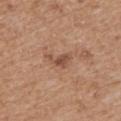Impression:
Captured during whole-body skin photography for melanoma surveillance; the lesion was not biopsied.
Clinical summary:
This is a white-light tile. Automated tile analysis of the lesion measured border irregularity of about 5.5 on a 0–10 scale and a peripheral color-asymmetry measure near 0.5. A male patient aged 68–72. Measured at roughly 3 mm in maximum diameter. A 15 mm close-up tile from a total-body photography series done for melanoma screening. Located on the mid back.